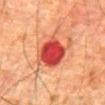Imaged during a routine full-body skin examination; the lesion was not biopsied and no histopathology is available. The lesion is on the abdomen. The recorded lesion diameter is about 4 mm. A male patient, in their 80s. A 15 mm crop from a total-body photograph taken for skin-cancer surveillance. Automated tile analysis of the lesion measured border irregularity of about 1.5 on a 0–10 scale, internal color variation of about 4.5 on a 0–10 scale, and peripheral color asymmetry of about 1.5. The software also gave a nevus-likeness score of about 0/100. This is a cross-polarized tile.